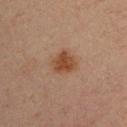Q: Was this lesion biopsied?
A: total-body-photography surveillance lesion; no biopsy
Q: Patient demographics?
A: male, aged around 30
Q: What is the anatomic site?
A: the left upper arm
Q: Illumination type?
A: cross-polarized illumination
Q: Automated lesion metrics?
A: a lesion color around L≈39 a*≈17 b*≈26 in CIELAB, roughly 7 lightness units darker than nearby skin, and a normalized lesion–skin contrast near 7; a border-irregularity rating of about 1.5/10 and internal color variation of about 3.5 on a 0–10 scale
Q: How was this image acquired?
A: ~15 mm crop, total-body skin-cancer survey
Q: How large is the lesion?
A: ~4 mm (longest diameter)